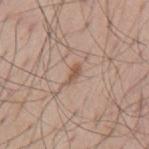Captured during whole-body skin photography for melanoma surveillance; the lesion was not biopsied.